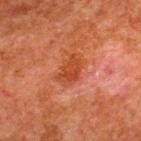acquisition: ~15 mm tile from a whole-body skin photo
diameter: ≈3.5 mm
body site: the upper back
automated lesion analysis: a lesion color around L≈36 a*≈28 b*≈33 in CIELAB, a lesion–skin lightness drop of about 7, and a normalized border contrast of about 7; a nevus-likeness score of about 0/100
subject: male, in their 80s